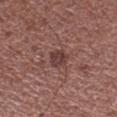{"biopsy_status": "not biopsied; imaged during a skin examination", "lighting": "white-light", "image": {"source": "total-body photography crop", "field_of_view_mm": 15}, "lesion_size": {"long_diameter_mm_approx": 2.5}, "patient": {"sex": "male", "age_approx": 65}, "site": "right lower leg"}